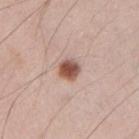Q: Is there a histopathology result?
A: imaged on a skin check; not biopsied
Q: What did automated image analysis measure?
A: an area of roughly 5 mm², an outline eccentricity of about 0.35 (0 = round, 1 = elongated), and a symmetry-axis asymmetry near 0.15
Q: What is the anatomic site?
A: the arm
Q: Illumination type?
A: white-light
Q: What kind of image is this?
A: total-body-photography crop, ~15 mm field of view
Q: Patient demographics?
A: male, in their mid-30s
Q: Lesion size?
A: ≈2.5 mm